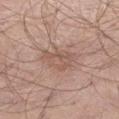biopsy_status: not biopsied; imaged during a skin examination
lesion_size:
  long_diameter_mm_approx: 4.0
site: left thigh
lighting: white-light
image:
  source: total-body photography crop
  field_of_view_mm: 15
patient:
  sex: male
  age_approx: 70